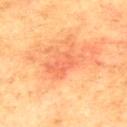  biopsy_status: not biopsied; imaged during a skin examination
  patient:
    sex: male
    age_approx: 75
  image:
    source: total-body photography crop
    field_of_view_mm: 15
  site: upper back
  lighting: cross-polarized